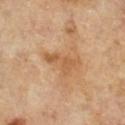| key | value |
|---|---|
| workup | catalogued during a skin exam; not biopsied |
| automated lesion analysis | a footprint of about 6.5 mm², a shape eccentricity near 0.9, and a shape-asymmetry score of about 0.5 (0 = symmetric); about 8 CIELAB-L* units darker than the surrounding skin and a lesion-to-skin contrast of about 6.5 (normalized; higher = more distinct); a border-irregularity rating of about 6/10, a within-lesion color-variation index near 2/10, and a peripheral color-asymmetry measure near 0.5 |
| image | 15 mm crop, total-body photography |
| lesion size | ≈4.5 mm |
| body site | the right lower leg |
| subject | male, aged 63 to 67 |
| tile lighting | cross-polarized |